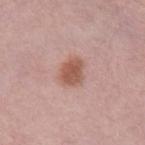No biopsy was performed on this lesion — it was imaged during a full skin examination and was not determined to be concerning. On the chest. The recorded lesion diameter is about 3.5 mm. This is a white-light tile. A 15 mm crop from a total-body photograph taken for skin-cancer surveillance. A female patient aged approximately 65. The total-body-photography lesion software estimated a lesion color around L≈55 a*≈23 b*≈27 in CIELAB.